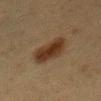follow-up = imaged on a skin check; not biopsied | location = the abdomen | patient = female, in their 40s | image = ~15 mm crop, total-body skin-cancer survey | image-analysis metrics = a detector confidence of about 100 out of 100 that the crop contains a lesion | lesion diameter = ≈5.5 mm.